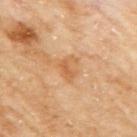biopsy_status: not biopsied; imaged during a skin examination
site: right upper arm
image:
  source: total-body photography crop
  field_of_view_mm: 15
lesion_size:
  long_diameter_mm_approx: 3.0
lighting: cross-polarized
patient:
  sex: female
  age_approx: 60